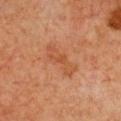{
  "biopsy_status": "not biopsied; imaged during a skin examination",
  "automated_metrics": {
    "area_mm2_approx": 8.0,
    "color_variation_0_10": 2.5,
    "peripheral_color_asymmetry": 0.5,
    "nevus_likeness_0_100": 0
  },
  "lesion_size": {
    "long_diameter_mm_approx": 5.0
  },
  "image": {
    "source": "total-body photography crop",
    "field_of_view_mm": 15
  },
  "site": "chest",
  "patient": {
    "sex": "female",
    "age_approx": 40
  },
  "lighting": "cross-polarized"
}